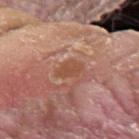Impression:
Part of a total-body skin-imaging series; this lesion was reviewed on a skin check and was not flagged for biopsy.
Image and clinical context:
The lesion is on the left forearm. A male patient about 40 years old. A close-up tile cropped from a whole-body skin photograph, about 15 mm across.